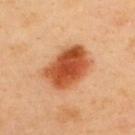follow-up: imaged on a skin check; not biopsied
tile lighting: cross-polarized illumination
imaging modality: ~15 mm crop, total-body skin-cancer survey
patient: male, about 40 years old
TBP lesion metrics: an area of roughly 20 mm², an outline eccentricity of about 0.7 (0 = round, 1 = elongated), and two-axis asymmetry of about 0.15; a mean CIELAB color near L≈54 a*≈31 b*≈42 and a lesion-to-skin contrast of about 11 (normalized; higher = more distinct)
location: the upper back
lesion size: about 5.5 mm